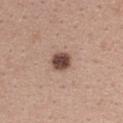Findings:
– image source · ~15 mm tile from a whole-body skin photo
– subject · female, roughly 40 years of age
– body site · the upper back